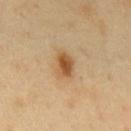Part of a total-body skin-imaging series; this lesion was reviewed on a skin check and was not flagged for biopsy. Longest diameter approximately 3 mm. Imaged with cross-polarized lighting. A male patient aged 48 to 52. Automated tile analysis of the lesion measured an average lesion color of about L≈53 a*≈19 b*≈39 (CIELAB) and a normalized lesion–skin contrast near 9. The analysis additionally found an automated nevus-likeness rating near 95 out of 100. A region of skin cropped from a whole-body photographic capture, roughly 15 mm wide. The lesion is located on the abdomen.